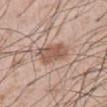No biopsy was performed on this lesion — it was imaged during a full skin examination and was not determined to be concerning. The subject is a male aged 53–57. Captured under white-light illumination. A lesion tile, about 15 mm wide, cut from a 3D total-body photograph. From the abdomen. Automated tile analysis of the lesion measured an area of roughly 10 mm², a shape eccentricity near 0.7, and two-axis asymmetry of about 0.2. The analysis additionally found a lesion-detection confidence of about 100/100.